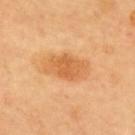Case summary:
– follow-up — imaged on a skin check; not biopsied
– site — the upper back
– acquisition — ~15 mm crop, total-body skin-cancer survey
– lesion size — ≈5 mm
– patient — male, aged approximately 55
– lighting — cross-polarized illumination